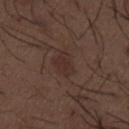Assessment: Recorded during total-body skin imaging; not selected for excision or biopsy. Clinical summary: A 15 mm crop from a total-body photograph taken for skin-cancer surveillance. An algorithmic analysis of the crop reported an automated nevus-likeness rating near 20 out of 100. The lesion is on the mid back. Approximately 3.5 mm at its widest. The subject is a male about 50 years old.